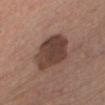No biopsy was performed on this lesion — it was imaged during a full skin examination and was not determined to be concerning. The lesion is on the chest. A lesion tile, about 15 mm wide, cut from a 3D total-body photograph. A male subject, aged 58 to 62.